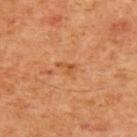<lesion>
<biopsy_status>not biopsied; imaged during a skin examination</biopsy_status>
<patient>
  <sex>male</sex>
  <age_approx>60</age_approx>
</patient>
<site>chest</site>
<lighting>cross-polarized</lighting>
<lesion_size>
  <long_diameter_mm_approx>2.5</long_diameter_mm_approx>
</lesion_size>
<image>
  <source>total-body photography crop</source>
  <field_of_view_mm>15</field_of_view_mm>
</image>
</lesion>